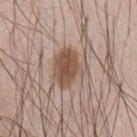{"biopsy_status": "not biopsied; imaged during a skin examination", "lesion_size": {"long_diameter_mm_approx": 4.0}, "automated_metrics": {"nevus_likeness_0_100": 100}, "lighting": "white-light", "image": {"source": "total-body photography crop", "field_of_view_mm": 15}, "patient": {"sex": "male", "age_approx": 55}}